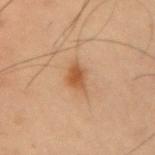This lesion was catalogued during total-body skin photography and was not selected for biopsy. Approximately 4 mm at its widest. An algorithmic analysis of the crop reported an average lesion color of about L≈44 a*≈18 b*≈31 (CIELAB) and roughly 9 lightness units darker than nearby skin. And it measured a border-irregularity rating of about 3.5/10 and a peripheral color-asymmetry measure near 0.5. Captured under cross-polarized illumination. Located on the arm. Cropped from a whole-body photographic skin survey; the tile spans about 15 mm. The patient is a male aged 53 to 57.